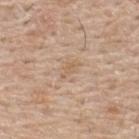Notes:
– image source · ~15 mm tile from a whole-body skin photo
– size · ≈2.5 mm
– patient · male, aged 53–57
– body site · the upper back
– lighting · white-light